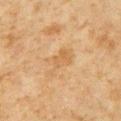Clinical impression:
The lesion was tiled from a total-body skin photograph and was not biopsied.
Acquisition and patient details:
About 4 mm across. Cropped from a whole-body photographic skin survey; the tile spans about 15 mm. Imaged with cross-polarized lighting. A male patient, in their 60s. The lesion is on the left upper arm.